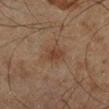No biopsy was performed on this lesion — it was imaged during a full skin examination and was not determined to be concerning. The lesion-visualizer software estimated a border-irregularity index near 3/10, a color-variation rating of about 2/10, and peripheral color asymmetry of about 0.5. Imaged with cross-polarized lighting. A male patient, in their mid-60s. The lesion is on the left lower leg. This image is a 15 mm lesion crop taken from a total-body photograph. About 3 mm across.